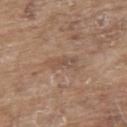Imaged during a routine full-body skin examination; the lesion was not biopsied and no histopathology is available.
The patient is a male in their 80s.
A 15 mm close-up extracted from a 3D total-body photography capture.
Located on the upper back.